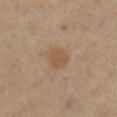Imaged during a routine full-body skin examination; the lesion was not biopsied and no histopathology is available.
Approximately 2.5 mm at its widest.
The lesion is located on the right lower leg.
Captured under cross-polarized illumination.
This image is a 15 mm lesion crop taken from a total-body photograph.
The patient is a female in their mid- to late 40s.